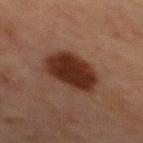workup: catalogued during a skin exam; not biopsied
site: the front of the torso
imaging modality: total-body-photography crop, ~15 mm field of view
patient: male, about 65 years old
illumination: cross-polarized illumination